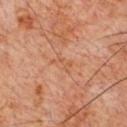No biopsy was performed on this lesion — it was imaged during a full skin examination and was not determined to be concerning.
The recorded lesion diameter is about 2.5 mm.
Captured under cross-polarized illumination.
From the front of the torso.
A region of skin cropped from a whole-body photographic capture, roughly 15 mm wide.
A male patient, aged 58 to 62.